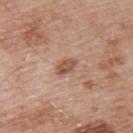The lesion was tiled from a total-body skin photograph and was not biopsied. A male patient, approximately 55 years of age. This image is a 15 mm lesion crop taken from a total-body photograph. The lesion is on the upper back.